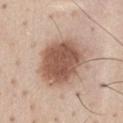The lesion was photographed on a routine skin check and not biopsied; there is no pathology result. Imaged with white-light lighting. From the chest. A male subject in their mid-40s. Cropped from a total-body skin-imaging series; the visible field is about 15 mm. The total-body-photography lesion software estimated a border-irregularity rating of about 2/10, a color-variation rating of about 4/10, and a peripheral color-asymmetry measure near 1. About 6.5 mm across.